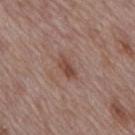Q: Was this lesion biopsied?
A: total-body-photography surveillance lesion; no biopsy
Q: What did automated image analysis measure?
A: an outline eccentricity of about 0.9 (0 = round, 1 = elongated); a lesion color around L≈46 a*≈20 b*≈25 in CIELAB and roughly 9 lightness units darker than nearby skin
Q: Illumination type?
A: white-light
Q: Who is the patient?
A: male, roughly 70 years of age
Q: What is the lesion's diameter?
A: about 3 mm
Q: What is the imaging modality?
A: 15 mm crop, total-body photography
Q: Lesion location?
A: the back